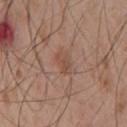No biopsy was performed on this lesion — it was imaged during a full skin examination and was not determined to be concerning. The lesion's longest dimension is about 2.5 mm. This image is a 15 mm lesion crop taken from a total-body photograph. The lesion is on the chest. Automated image analysis of the tile measured a lesion area of about 3 mm² and a shape eccentricity near 0.8. And it measured a nevus-likeness score of about 5/100 and lesion-presence confidence of about 100/100. The subject is a male aged approximately 55.